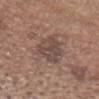automated metrics — an eccentricity of roughly 0.75 and a symmetry-axis asymmetry near 0.3; a border-irregularity rating of about 3.5/10 and a color-variation rating of about 4/10 | anatomic site — the head or neck | tile lighting — white-light illumination | diameter — ~5 mm (longest diameter) | subject — male, aged around 65 | image source — 15 mm crop, total-body photography.